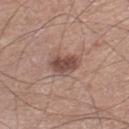The lesion was tiled from a total-body skin photograph and was not biopsied.